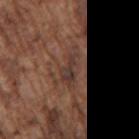Assessment:
Recorded during total-body skin imaging; not selected for excision or biopsy.
Context:
The subject is a male in their mid- to late 70s. A close-up tile cropped from a whole-body skin photograph, about 15 mm across. From the right upper arm.